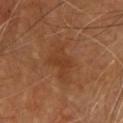| field | value |
|---|---|
| follow-up | total-body-photography surveillance lesion; no biopsy |
| size | about 5.5 mm |
| patient | male, aged around 55 |
| acquisition | 15 mm crop, total-body photography |
| site | the chest |
| tile lighting | cross-polarized illumination |